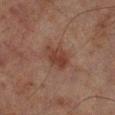Imaged during a routine full-body skin examination; the lesion was not biopsied and no histopathology is available.
Measured at roughly 4 mm in maximum diameter.
A male subject, aged 68 to 72.
Cropped from a total-body skin-imaging series; the visible field is about 15 mm.
Located on the right lower leg.
Automated image analysis of the tile measured a footprint of about 8.5 mm², a shape eccentricity near 0.75, and a symmetry-axis asymmetry near 0.3. The analysis additionally found an average lesion color of about L≈32 a*≈18 b*≈22 (CIELAB) and a normalized lesion–skin contrast near 7. It also reported an automated nevus-likeness rating near 25 out of 100.
Captured under cross-polarized illumination.